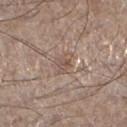biopsy status: imaged on a skin check; not biopsied
imaging modality: 15 mm crop, total-body photography
subject: male, about 60 years old
site: the left lower leg
lesion diameter: ≈3 mm